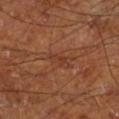Case summary:
* follow-up — no biopsy performed (imaged during a skin exam)
* patient — male, about 65 years old
* anatomic site — the right lower leg
* image-analysis metrics — an average lesion color of about L≈35 a*≈22 b*≈29 (CIELAB), about 6 CIELAB-L* units darker than the surrounding skin, and a normalized border contrast of about 5.5; a classifier nevus-likeness of about 0/100 and lesion-presence confidence of about 50/100
* image source — total-body-photography crop, ~15 mm field of view
* tile lighting — cross-polarized
* lesion size — ≈3 mm